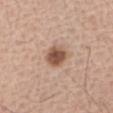notes — total-body-photography surveillance lesion; no biopsy
body site — the right forearm
patient — male, in their 60s
acquisition — ~15 mm tile from a whole-body skin photo